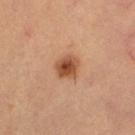This lesion was catalogued during total-body skin photography and was not selected for biopsy.
A female subject aged approximately 30.
About 3 mm across.
A 15 mm crop from a total-body photograph taken for skin-cancer surveillance.
The lesion is located on the left thigh.
This is a cross-polarized tile.